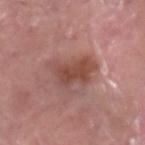An algorithmic analysis of the crop reported a lesion-detection confidence of about 100/100.
On the right lower leg.
A male subject aged around 40.
The tile uses white-light illumination.
A lesion tile, about 15 mm wide, cut from a 3D total-body photograph.
About 5.5 mm across.